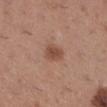biopsy_status: not biopsied; imaged during a skin examination
image:
  source: total-body photography crop
  field_of_view_mm: 15
lighting: white-light
site: right lower leg
patient:
  sex: female
  age_approx: 30
lesion_size:
  long_diameter_mm_approx: 3.0
automated_metrics:
  cielab_L: 49
  cielab_a: 22
  cielab_b: 28
  vs_skin_contrast_norm: 7.5
  border_irregularity_0_10: 2.0
  peripheral_color_asymmetry: 0.5